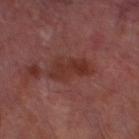Clinical impression: The lesion was photographed on a routine skin check and not biopsied; there is no pathology result. Clinical summary: A male subject, approximately 70 years of age. On the left lower leg. Cropped from a whole-body photographic skin survey; the tile spans about 15 mm. The recorded lesion diameter is about 4.5 mm. Captured under cross-polarized illumination.